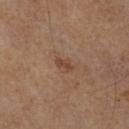The lesion was photographed on a routine skin check and not biopsied; there is no pathology result.